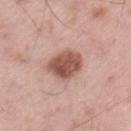This lesion was catalogued during total-body skin photography and was not selected for biopsy. The lesion-visualizer software estimated a lesion area of about 11 mm², an outline eccentricity of about 0.65 (0 = round, 1 = elongated), and two-axis asymmetry of about 0.15. The analysis additionally found a mean CIELAB color near L≈54 a*≈22 b*≈26, roughly 15 lightness units darker than nearby skin, and a lesion-to-skin contrast of about 10 (normalized; higher = more distinct). And it measured a nevus-likeness score of about 70/100 and a lesion-detection confidence of about 100/100. The recorded lesion diameter is about 4.5 mm. A male subject, about 55 years old. A 15 mm crop from a total-body photograph taken for skin-cancer surveillance. On the left thigh.